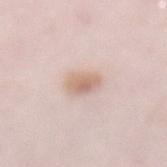follow-up — catalogued during a skin exam; not biopsied
diameter — ~3 mm (longest diameter)
body site — the front of the torso
patient — female, aged approximately 65
imaging modality — 15 mm crop, total-body photography
lighting — white-light illumination
automated metrics — an area of roughly 6.5 mm², a shape eccentricity near 0.65, and a shape-asymmetry score of about 0.15 (0 = symmetric); a mean CIELAB color near L≈68 a*≈16 b*≈27 and a lesion–skin lightness drop of about 10; an automated nevus-likeness rating near 85 out of 100 and a lesion-detection confidence of about 100/100